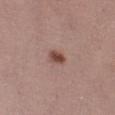The lesion was tiled from a total-body skin photograph and was not biopsied. A lesion tile, about 15 mm wide, cut from a 3D total-body photograph. Imaged with white-light lighting. A female subject, aged approximately 30. The lesion is located on the left thigh. Longest diameter approximately 2.5 mm. The total-body-photography lesion software estimated a lesion area of about 3 mm², an outline eccentricity of about 0.75 (0 = round, 1 = elongated), and two-axis asymmetry of about 0.2. The software also gave a lesion color around L≈46 a*≈21 b*≈24 in CIELAB and a lesion–skin lightness drop of about 13. The software also gave a within-lesion color-variation index near 2/10 and peripheral color asymmetry of about 1. The software also gave a nevus-likeness score of about 95/100 and a lesion-detection confidence of about 100/100.